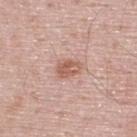Q: Is there a histopathology result?
A: catalogued during a skin exam; not biopsied